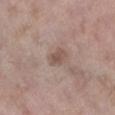follow-up: no biopsy performed (imaged during a skin exam).